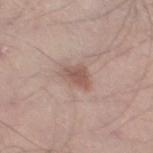No biopsy was performed on this lesion — it was imaged during a full skin examination and was not determined to be concerning.
A male subject in their mid- to late 50s.
Located on the left thigh.
A lesion tile, about 15 mm wide, cut from a 3D total-body photograph.
Imaged with white-light lighting.
The lesion's longest dimension is about 3.5 mm.